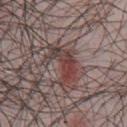biopsy status = catalogued during a skin exam; not biopsied
TBP lesion metrics = a footprint of about 12 mm² and two-axis asymmetry of about 0.6; an average lesion color of about L≈41 a*≈18 b*≈19 (CIELAB), about 9 CIELAB-L* units darker than the surrounding skin, and a normalized border contrast of about 8
lighting = white-light illumination
patient = male, aged 38 to 42
imaging modality = ~15 mm crop, total-body skin-cancer survey
location = the chest